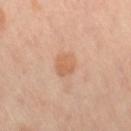anatomic site: the right thigh
size: about 2.5 mm
illumination: cross-polarized
image-analysis metrics: a mean CIELAB color near L≈63 a*≈23 b*≈35, roughly 8 lightness units darker than nearby skin, and a lesion-to-skin contrast of about 6 (normalized; higher = more distinct); border irregularity of about 1.5 on a 0–10 scale and a color-variation rating of about 1.5/10
patient: female, roughly 50 years of age
image: total-body-photography crop, ~15 mm field of view